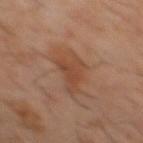follow-up: catalogued during a skin exam; not biopsied | lesion size: ~5.5 mm (longest diameter) | acquisition: ~15 mm crop, total-body skin-cancer survey | lighting: cross-polarized | location: the mid back | patient: male, roughly 60 years of age.